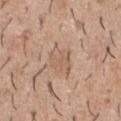| field | value |
|---|---|
| workup | catalogued during a skin exam; not biopsied |
| site | the chest |
| automated metrics | a lesion area of about 7.5 mm², an eccentricity of roughly 0.75, and a shape-asymmetry score of about 0.2 (0 = symmetric); an average lesion color of about L≈59 a*≈17 b*≈29 (CIELAB), a lesion–skin lightness drop of about 7, and a lesion-to-skin contrast of about 4.5 (normalized; higher = more distinct) |
| image source | ~15 mm tile from a whole-body skin photo |
| patient | male, about 40 years old |
| lighting | white-light |